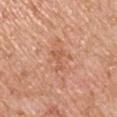The subject is a male roughly 60 years of age. The lesion is on the left upper arm. This is a white-light tile. A 15 mm crop from a total-body photograph taken for skin-cancer surveillance.